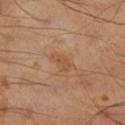No biopsy was performed on this lesion — it was imaged during a full skin examination and was not determined to be concerning. Captured under cross-polarized illumination. Automated image analysis of the tile measured a lesion area of about 4 mm², an eccentricity of roughly 0.85, and two-axis asymmetry of about 0.3. The software also gave an average lesion color of about L≈51 a*≈20 b*≈34 (CIELAB), about 6 CIELAB-L* units darker than the surrounding skin, and a normalized border contrast of about 5. A male subject in their mid- to late 40s. A 15 mm crop from a total-body photograph taken for skin-cancer surveillance. The lesion is located on the leg. About 3 mm across.